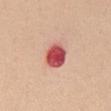The lesion was tiled from a total-body skin photograph and was not biopsied.
Captured under white-light illumination.
The subject is a female aged around 45.
A 15 mm close-up tile from a total-body photography series done for melanoma screening.
Located on the abdomen.
An algorithmic analysis of the crop reported a footprint of about 8 mm², an outline eccentricity of about 0.55 (0 = round, 1 = elongated), and a symmetry-axis asymmetry near 0.1. The analysis additionally found a lesion color around L≈53 a*≈38 b*≈27 in CIELAB, roughly 20 lightness units darker than nearby skin, and a normalized border contrast of about 12.5. It also reported border irregularity of about 1 on a 0–10 scale and a peripheral color-asymmetry measure near 1.5. The analysis additionally found an automated nevus-likeness rating near 0 out of 100 and a detector confidence of about 100 out of 100 that the crop contains a lesion.
Longest diameter approximately 3.5 mm.